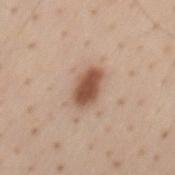| feature | finding |
|---|---|
| follow-up | total-body-photography surveillance lesion; no biopsy |
| anatomic site | the mid back |
| lesion size | about 4 mm |
| automated metrics | a footprint of about 8 mm², an eccentricity of roughly 0.8, and two-axis asymmetry of about 0.15; a lesion color around L≈53 a*≈20 b*≈30 in CIELAB, a lesion–skin lightness drop of about 15, and a normalized lesion–skin contrast near 10.5 |
| patient | male, aged approximately 60 |
| tile lighting | white-light |
| imaging modality | total-body-photography crop, ~15 mm field of view |